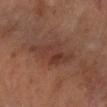Q: Was a biopsy performed?
A: catalogued during a skin exam; not biopsied
Q: What is the anatomic site?
A: the left forearm
Q: What kind of image is this?
A: 15 mm crop, total-body photography
Q: How was the tile lit?
A: cross-polarized illumination
Q: What is the lesion's diameter?
A: ≈7 mm
Q: Patient demographics?
A: male, aged approximately 55